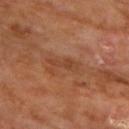{"biopsy_status": "not biopsied; imaged during a skin examination", "site": "chest", "image": {"source": "total-body photography crop", "field_of_view_mm": 15}, "patient": {"sex": "male", "age_approx": 60}}